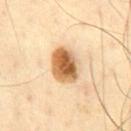Assessment:
Recorded during total-body skin imaging; not selected for excision or biopsy.
Context:
A 15 mm close-up tile from a total-body photography series done for melanoma screening. Automated tile analysis of the lesion measured a mean CIELAB color near L≈63 a*≈20 b*≈41 and a lesion–skin lightness drop of about 19. On the abdomen. Imaged with cross-polarized lighting. A male subject, in their mid- to late 60s.